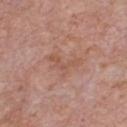{"biopsy_status": "not biopsied; imaged during a skin examination", "image": {"source": "total-body photography crop", "field_of_view_mm": 15}, "automated_metrics": {"border_irregularity_0_10": 8.0, "color_variation_0_10": 0.0, "peripheral_color_asymmetry": 0.0}, "patient": {"sex": "male", "age_approx": 60}, "lighting": "white-light", "site": "chest"}